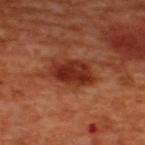biopsy_status: not biopsied; imaged during a skin examination
lesion_size:
  long_diameter_mm_approx: 5.5
patient:
  sex: male
  age_approx: 50
site: upper back
lighting: cross-polarized
image:
  source: total-body photography crop
  field_of_view_mm: 15